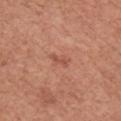{
  "biopsy_status": "not biopsied; imaged during a skin examination",
  "patient": {
    "sex": "male",
    "age_approx": 75
  },
  "site": "chest",
  "image": {
    "source": "total-body photography crop",
    "field_of_view_mm": 15
  },
  "lesion_size": {
    "long_diameter_mm_approx": 2.5
  },
  "automated_metrics": {
    "nevus_likeness_0_100": 0,
    "lesion_detection_confidence_0_100": 100
  },
  "lighting": "white-light"
}